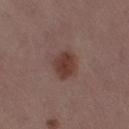Impression: Part of a total-body skin-imaging series; this lesion was reviewed on a skin check and was not flagged for biopsy. Background: The tile uses white-light illumination. This image is a 15 mm lesion crop taken from a total-body photograph. The lesion-visualizer software estimated a shape eccentricity near 0.6 and a symmetry-axis asymmetry near 0.2. And it measured a nevus-likeness score of about 95/100 and a detector confidence of about 100 out of 100 that the crop contains a lesion. The lesion is located on the right thigh. The patient is a female about 55 years old.